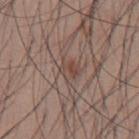The lesion was photographed on a routine skin check and not biopsied; there is no pathology result.
A 15 mm close-up extracted from a 3D total-body photography capture.
Measured at roughly 3 mm in maximum diameter.
The lesion-visualizer software estimated an area of roughly 5.5 mm², a shape eccentricity near 0.6, and two-axis asymmetry of about 0.3.
The patient is a male approximately 35 years of age.
The lesion is located on the abdomen.
Captured under white-light illumination.